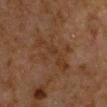Recorded during total-body skin imaging; not selected for excision or biopsy. Automated tile analysis of the lesion measured a lesion area of about 12 mm², an eccentricity of roughly 0.85, and a symmetry-axis asymmetry near 0.45. The software also gave a lesion color around L≈28 a*≈15 b*≈25 in CIELAB, about 4 CIELAB-L* units darker than the surrounding skin, and a lesion-to-skin contrast of about 5 (normalized; higher = more distinct). The software also gave a nevus-likeness score of about 0/100 and lesion-presence confidence of about 95/100. The tile uses cross-polarized illumination. A 15 mm close-up extracted from a 3D total-body photography capture. A male subject, roughly 50 years of age. Longest diameter approximately 6 mm. From the left upper arm.